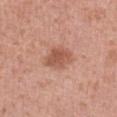The tile uses white-light illumination.
The total-body-photography lesion software estimated border irregularity of about 2 on a 0–10 scale. And it measured a nevus-likeness score of about 75/100 and a detector confidence of about 100 out of 100 that the crop contains a lesion.
A female subject roughly 40 years of age.
The recorded lesion diameter is about 3.5 mm.
This image is a 15 mm lesion crop taken from a total-body photograph.
On the left forearm.